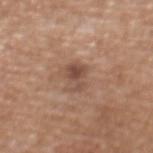• biopsy status — total-body-photography surveillance lesion; no biopsy
• lighting — white-light
• patient — female, aged around 70
• site — the upper back
• size — ~3 mm (longest diameter)
• image source — ~15 mm tile from a whole-body skin photo